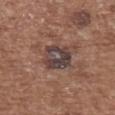Image and clinical context:
A female subject aged around 75. A roughly 15 mm field-of-view crop from a total-body skin photograph. On the upper back.